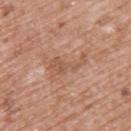The total-body-photography lesion software estimated an average lesion color of about L≈55 a*≈21 b*≈31 (CIELAB), about 7 CIELAB-L* units darker than the surrounding skin, and a normalized lesion–skin contrast near 5.5. The analysis additionally found a classifier nevus-likeness of about 0/100 and a detector confidence of about 100 out of 100 that the crop contains a lesion. Located on the upper back. A female patient aged 38 to 42. The recorded lesion diameter is about 5 mm. A 15 mm close-up extracted from a 3D total-body photography capture. Imaged with white-light lighting.